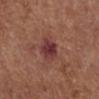• workup — catalogued during a skin exam; not biopsied
• subject — male, roughly 75 years of age
• image source — ~15 mm tile from a whole-body skin photo
• TBP lesion metrics — a footprint of about 6 mm², an eccentricity of roughly 0.25, and a shape-asymmetry score of about 0.2 (0 = symmetric); a border-irregularity index near 1.5/10, a within-lesion color-variation index near 4/10, and peripheral color asymmetry of about 1.5; a nevus-likeness score of about 20/100 and lesion-presence confidence of about 100/100
• lesion size — ~3 mm (longest diameter)
• site — the right lower leg
• lighting — white-light